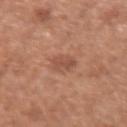Imaged during a routine full-body skin examination; the lesion was not biopsied and no histopathology is available. A 15 mm crop from a total-body photograph taken for skin-cancer surveillance. On the right upper arm. The subject is a female about 40 years old.